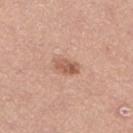Impression: This lesion was catalogued during total-body skin photography and was not selected for biopsy. Context: Captured under white-light illumination. The subject is a female aged 33–37. From the left lower leg. A region of skin cropped from a whole-body photographic capture, roughly 15 mm wide.